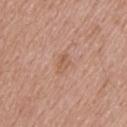The lesion was photographed on a routine skin check and not biopsied; there is no pathology result. Cropped from a total-body skin-imaging series; the visible field is about 15 mm. The patient is a male aged approximately 55. Longest diameter approximately 2.5 mm. Captured under white-light illumination. On the upper back.